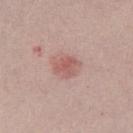No biopsy was performed on this lesion — it was imaged during a full skin examination and was not determined to be concerning.
A 15 mm close-up tile from a total-body photography series done for melanoma screening.
The total-body-photography lesion software estimated an area of roughly 5.5 mm², an eccentricity of roughly 0.6, and a symmetry-axis asymmetry near 0.2. The software also gave an average lesion color of about L≈57 a*≈24 b*≈23 (CIELAB), about 9 CIELAB-L* units darker than the surrounding skin, and a lesion-to-skin contrast of about 6.5 (normalized; higher = more distinct).
A male subject, about 30 years old.
Longest diameter approximately 3 mm.